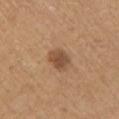– biopsy status — imaged on a skin check; not biopsied
– lesion size — about 3 mm
– imaging modality — ~15 mm crop, total-body skin-cancer survey
– subject — male, aged around 65
– location — the left upper arm